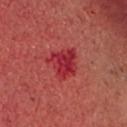Findings:
* follow-up — no biopsy performed (imaged during a skin exam)
* patient — male, roughly 65 years of age
* imaging modality — ~15 mm crop, total-body skin-cancer survey
* lesion size — ~4 mm (longest diameter)
* body site — the head or neck